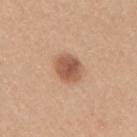Clinical impression: Recorded during total-body skin imaging; not selected for excision or biopsy. Acquisition and patient details: Captured under white-light illumination. The lesion-visualizer software estimated a lesion area of about 8.5 mm², a shape eccentricity near 0.45, and a shape-asymmetry score of about 0.15 (0 = symmetric). And it measured a mean CIELAB color near L≈56 a*≈22 b*≈31, about 13 CIELAB-L* units darker than the surrounding skin, and a lesion-to-skin contrast of about 8.5 (normalized; higher = more distinct). The software also gave border irregularity of about 1.5 on a 0–10 scale, internal color variation of about 4 on a 0–10 scale, and peripheral color asymmetry of about 1. The software also gave a detector confidence of about 100 out of 100 that the crop contains a lesion. The lesion is on the arm. Cropped from a total-body skin-imaging series; the visible field is about 15 mm. A female patient in their 40s. The recorded lesion diameter is about 3.5 mm.